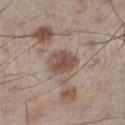Assessment:
No biopsy was performed on this lesion — it was imaged during a full skin examination and was not determined to be concerning.
Background:
The lesion is on the left lower leg. Longest diameter approximately 4 mm. The subject is a male aged around 60. A roughly 15 mm field-of-view crop from a total-body skin photograph. An algorithmic analysis of the crop reported a footprint of about 9 mm², a shape eccentricity near 0.7, and two-axis asymmetry of about 0.15. It also reported an average lesion color of about L≈50 a*≈17 b*≈24 (CIELAB) and roughly 11 lightness units darker than nearby skin. It also reported a border-irregularity index near 1.5/10, a within-lesion color-variation index near 4/10, and peripheral color asymmetry of about 1.5. Captured under white-light illumination.